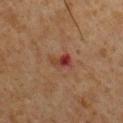biopsy_status: not biopsied; imaged during a skin examination
patient:
  sex: male
  age_approx: 60
site: chest
automated_metrics:
  cielab_L: 32
  cielab_a: 24
  cielab_b: 25
  vs_skin_darker_L: 9.0
  vs_skin_contrast_norm: 8.5
  lesion_detection_confidence_0_100: 100
image:
  source: total-body photography crop
  field_of_view_mm: 15
lighting: cross-polarized
lesion_size:
  long_diameter_mm_approx: 2.5